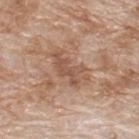  biopsy_status: not biopsied; imaged during a skin examination
  site: upper back
  patient:
    sex: male
    age_approx: 80
  lesion_size:
    long_diameter_mm_approx: 5.0
  image:
    source: total-body photography crop
    field_of_view_mm: 15